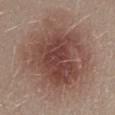{"biopsy_status": "not biopsied; imaged during a skin examination", "patient": {"sex": "male", "age_approx": 30}, "lighting": "white-light", "lesion_size": {"long_diameter_mm_approx": 11.0}, "site": "mid back", "automated_metrics": {"area_mm2_approx": 60.0, "shape_asymmetry": 0.15, "border_irregularity_0_10": 3.5, "color_variation_0_10": 6.5, "peripheral_color_asymmetry": 2.0}, "image": {"source": "total-body photography crop", "field_of_view_mm": 15}}